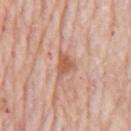follow-up = catalogued during a skin exam; not biopsied | image = total-body-photography crop, ~15 mm field of view | body site = the front of the torso | lesion diameter = ≈3.5 mm | subject = male, roughly 80 years of age | lighting = white-light illumination.